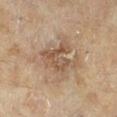The lesion was photographed on a routine skin check and not biopsied; there is no pathology result. On the right lower leg. A roughly 15 mm field-of-view crop from a total-body skin photograph. A female patient in their 60s. Approximately 5 mm at its widest. This is a cross-polarized tile.